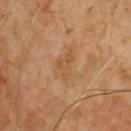| feature | finding |
|---|---|
| image source | ~15 mm tile from a whole-body skin photo |
| lesion diameter | ~3 mm (longest diameter) |
| subject | male, approximately 65 years of age |
| tile lighting | cross-polarized |